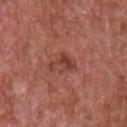* notes · total-body-photography surveillance lesion; no biopsy
* patient · male, in their mid- to late 60s
* image · ~15 mm crop, total-body skin-cancer survey
* body site · the chest
* automated lesion analysis · a lesion area of about 5 mm², an outline eccentricity of about 0.85 (0 = round, 1 = elongated), and a symmetry-axis asymmetry near 0.5; an average lesion color of about L≈42 a*≈28 b*≈26 (CIELAB), about 9 CIELAB-L* units darker than the surrounding skin, and a normalized lesion–skin contrast near 7; border irregularity of about 7 on a 0–10 scale, internal color variation of about 1.5 on a 0–10 scale, and a peripheral color-asymmetry measure near 0.5; a nevus-likeness score of about 0/100 and a detector confidence of about 100 out of 100 that the crop contains a lesion
* lesion diameter · ~3.5 mm (longest diameter)